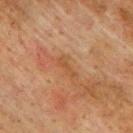{
  "biopsy_status": "not biopsied; imaged during a skin examination",
  "image": {
    "source": "total-body photography crop",
    "field_of_view_mm": 15
  },
  "lighting": "cross-polarized",
  "lesion_size": {
    "long_diameter_mm_approx": 3.0
  },
  "patient": {
    "sex": "male",
    "age_approx": 75
  },
  "site": "upper back"
}